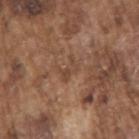No biopsy was performed on this lesion — it was imaged during a full skin examination and was not determined to be concerning.